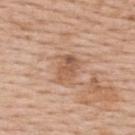The lesion was tiled from a total-body skin photograph and was not biopsied.